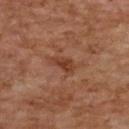Captured during whole-body skin photography for melanoma surveillance; the lesion was not biopsied. This is a cross-polarized tile. The lesion's longest dimension is about 3.5 mm. From the back. Cropped from a whole-body photographic skin survey; the tile spans about 15 mm. The lesion-visualizer software estimated a mean CIELAB color near L≈34 a*≈21 b*≈28, a lesion–skin lightness drop of about 7, and a normalized lesion–skin contrast near 7. The software also gave a border-irregularity rating of about 3/10 and a peripheral color-asymmetry measure near 0.5. The software also gave a classifier nevus-likeness of about 0/100 and a lesion-detection confidence of about 100/100. The subject is a female approximately 60 years of age.